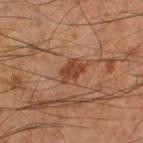The lesion was photographed on a routine skin check and not biopsied; there is no pathology result.
A 15 mm crop from a total-body photograph taken for skin-cancer surveillance.
From the right thigh.
A male patient aged approximately 60.